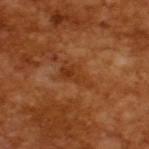biopsy status: catalogued during a skin exam; not biopsied
patient: male, about 65 years old
tile lighting: cross-polarized
image source: total-body-photography crop, ~15 mm field of view
lesion size: ≈4 mm